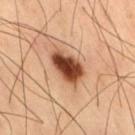Recorded during total-body skin imaging; not selected for excision or biopsy. About 4.5 mm across. On the right thigh. The patient is a male roughly 60 years of age. Imaged with cross-polarized lighting. A close-up tile cropped from a whole-body skin photograph, about 15 mm across.